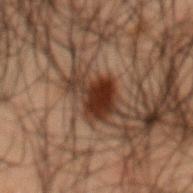Recorded during total-body skin imaging; not selected for excision or biopsy. Cropped from a total-body skin-imaging series; the visible field is about 15 mm. A male subject, aged 48 to 52. Located on the mid back.